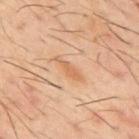acquisition = ~15 mm tile from a whole-body skin photo | subject = male, roughly 60 years of age | lesion diameter = about 4 mm | TBP lesion metrics = about 7 CIELAB-L* units darker than the surrounding skin and a normalized lesion–skin contrast near 5.5; a classifier nevus-likeness of about 0/100 and a lesion-detection confidence of about 100/100 | illumination = cross-polarized illumination | location = the upper back.